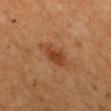Impression: The lesion was tiled from a total-body skin photograph and was not biopsied. Context: A 15 mm crop from a total-body photograph taken for skin-cancer surveillance. Automated tile analysis of the lesion measured a classifier nevus-likeness of about 65/100 and a lesion-detection confidence of about 100/100. The tile uses cross-polarized illumination. The lesion is on the back. Longest diameter approximately 5 mm. A male patient aged around 65.